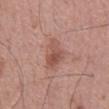This lesion was catalogued during total-body skin photography and was not selected for biopsy. Approximately 5 mm at its widest. Cropped from a whole-body photographic skin survey; the tile spans about 15 mm. The subject is a male in their mid- to late 50s. On the mid back. Captured under white-light illumination.